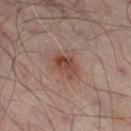biopsy status = catalogued during a skin exam; not biopsied
image = 15 mm crop, total-body photography
lesion diameter = about 3.5 mm
illumination = cross-polarized
body site = the right thigh
patient = male, in their mid- to late 60s